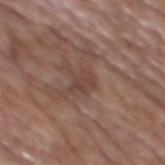<tbp_lesion>
<biopsy_status>not biopsied; imaged during a skin examination</biopsy_status>
<patient>
  <sex>male</sex>
  <age_approx>75</age_approx>
</patient>
<lesion_size>
  <long_diameter_mm_approx>3.0</long_diameter_mm_approx>
</lesion_size>
<image>
  <source>total-body photography crop</source>
  <field_of_view_mm>15</field_of_view_mm>
</image>
<automated_metrics>
  <area_mm2_approx>4.5</area_mm2_approx>
  <eccentricity>0.65</eccentricity>
  <shape_asymmetry>0.35</shape_asymmetry>
</automated_metrics>
<site>mid back</site>
</tbp_lesion>